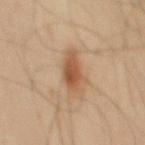The lesion was photographed on a routine skin check and not biopsied; there is no pathology result.
The subject is a male aged around 45.
The lesion is located on the mid back.
About 3.5 mm across.
A lesion tile, about 15 mm wide, cut from a 3D total-body photograph.